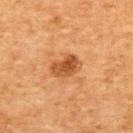Q: Was a biopsy performed?
A: total-body-photography surveillance lesion; no biopsy
Q: What are the patient's age and sex?
A: female, aged around 60
Q: What kind of image is this?
A: ~15 mm tile from a whole-body skin photo
Q: Where on the body is the lesion?
A: the back
Q: What lighting was used for the tile?
A: cross-polarized
Q: How large is the lesion?
A: about 3.5 mm